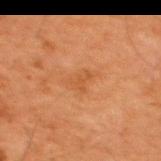Q: Was a biopsy performed?
A: catalogued during a skin exam; not biopsied
Q: Who is the patient?
A: male, aged 63 to 67
Q: Where on the body is the lesion?
A: the upper back
Q: Lesion size?
A: about 4 mm
Q: What lighting was used for the tile?
A: cross-polarized
Q: What did automated image analysis measure?
A: a border-irregularity index near 4.5/10, a within-lesion color-variation index near 1.5/10, and peripheral color asymmetry of about 0.5; a detector confidence of about 100 out of 100 that the crop contains a lesion
Q: How was this image acquired?
A: ~15 mm crop, total-body skin-cancer survey